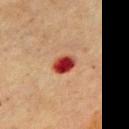No biopsy was performed on this lesion — it was imaged during a full skin examination and was not determined to be concerning.
Longest diameter approximately 2.5 mm.
The tile uses cross-polarized illumination.
A roughly 15 mm field-of-view crop from a total-body skin photograph.
The lesion-visualizer software estimated a lesion color around L≈39 a*≈36 b*≈31 in CIELAB. It also reported an automated nevus-likeness rating near 0 out of 100 and a detector confidence of about 100 out of 100 that the crop contains a lesion.
Located on the chest.
A female subject in their 60s.